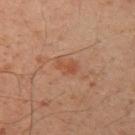| key | value |
|---|---|
| follow-up | total-body-photography surveillance lesion; no biopsy |
| subject | male, roughly 50 years of age |
| lighting | cross-polarized |
| imaging modality | ~15 mm crop, total-body skin-cancer survey |
| anatomic site | the left upper arm |
| lesion diameter | about 3 mm |